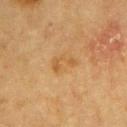| field | value |
|---|---|
| notes | total-body-photography surveillance lesion; no biopsy |
| patient | male, aged 83 or over |
| image | 15 mm crop, total-body photography |
| anatomic site | the chest |